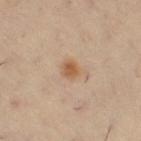Part of a total-body skin-imaging series; this lesion was reviewed on a skin check and was not flagged for biopsy.
The tile uses cross-polarized illumination.
The subject is a female in their mid- to late 40s.
A close-up tile cropped from a whole-body skin photograph, about 15 mm across.
Longest diameter approximately 2 mm.
From the left thigh.